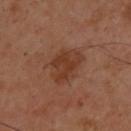Clinical summary: From the back. This image is a 15 mm lesion crop taken from a total-body photograph. The subject is a male about 40 years old. Measured at roughly 4.5 mm in maximum diameter.